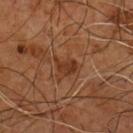tile lighting: cross-polarized illumination | imaging modality: total-body-photography crop, ~15 mm field of view | TBP lesion metrics: a lesion color around L≈32 a*≈21 b*≈30 in CIELAB and about 7 CIELAB-L* units darker than the surrounding skin; a border-irregularity index near 3.5/10, a within-lesion color-variation index near 2/10, and radial color variation of about 0.5; a detector confidence of about 100 out of 100 that the crop contains a lesion | body site: the chest | lesion size: ~3 mm (longest diameter) | patient: male, in their mid-50s.